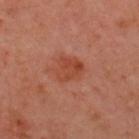Clinical impression: Imaged during a routine full-body skin examination; the lesion was not biopsied and no histopathology is available. Background: The tile uses cross-polarized illumination. The lesion's longest dimension is about 3 mm. A lesion tile, about 15 mm wide, cut from a 3D total-body photograph. The lesion-visualizer software estimated a footprint of about 6.5 mm² and a shape-asymmetry score of about 0.35 (0 = symmetric). The lesion is located on the upper back. The patient is a male roughly 50 years of age.